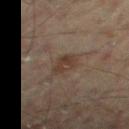- workup — catalogued during a skin exam; not biopsied
- body site — the leg
- subject — male, aged approximately 45
- illumination — cross-polarized illumination
- image source — total-body-photography crop, ~15 mm field of view